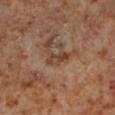| key | value |
|---|---|
| follow-up | imaged on a skin check; not biopsied |
| body site | the leg |
| imaging modality | total-body-photography crop, ~15 mm field of view |
| lesion diameter | about 3 mm |
| subject | male, about 60 years old |